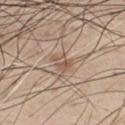Clinical impression:
No biopsy was performed on this lesion — it was imaged during a full skin examination and was not determined to be concerning.
Clinical summary:
Cropped from a total-body skin-imaging series; the visible field is about 15 mm. On the chest. The patient is a male approximately 45 years of age. The lesion's longest dimension is about 2.5 mm.